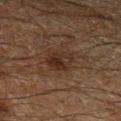This lesion was catalogued during total-body skin photography and was not selected for biopsy. The subject is a male about 60 years old. From the left lower leg. A 15 mm crop from a total-body photograph taken for skin-cancer surveillance.